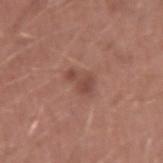Impression:
Captured during whole-body skin photography for melanoma surveillance; the lesion was not biopsied.
Background:
Cropped from a whole-body photographic skin survey; the tile spans about 15 mm. From the right upper arm. A male patient, aged 23–27. Captured under white-light illumination.